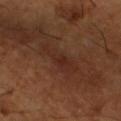This lesion was catalogued during total-body skin photography and was not selected for biopsy. The tile uses cross-polarized illumination. A lesion tile, about 15 mm wide, cut from a 3D total-body photograph. On the leg. Automated image analysis of the tile measured an area of roughly 7.5 mm². The software also gave a border-irregularity rating of about 5.5/10, internal color variation of about 2.5 on a 0–10 scale, and a peripheral color-asymmetry measure near 0.5. The analysis additionally found a classifier nevus-likeness of about 0/100 and a lesion-detection confidence of about 85/100. The subject is a male aged around 65.